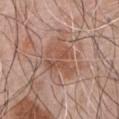Case summary:
• image source · 15 mm crop, total-body photography
• tile lighting · white-light illumination
• patient · male, approximately 70 years of age
• lesion size · about 5 mm
• body site · the chest
• image-analysis metrics · about 7 CIELAB-L* units darker than the surrounding skin and a lesion-to-skin contrast of about 6 (normalized; higher = more distinct)